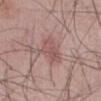workup — no biopsy performed (imaged during a skin exam); body site — the abdomen; subject — male, in their 60s; image source — ~15 mm tile from a whole-body skin photo; lighting — white-light; size — ≈3.5 mm.